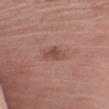This image is a 15 mm lesion crop taken from a total-body photograph.
Approximately 2.5 mm at its widest.
A female subject in their mid-70s.
Automated image analysis of the tile measured a border-irregularity index near 3/10. The analysis additionally found a nevus-likeness score of about 5/100 and a detector confidence of about 100 out of 100 that the crop contains a lesion.
The lesion is on the left upper arm.
The tile uses white-light illumination.